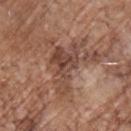Q: Was a biopsy performed?
A: catalogued during a skin exam; not biopsied
Q: Where on the body is the lesion?
A: the chest
Q: What are the patient's age and sex?
A: male, roughly 70 years of age
Q: How was this image acquired?
A: ~15 mm tile from a whole-body skin photo